The lesion is on the left lower leg. Cropped from a whole-body photographic skin survey; the tile spans about 15 mm. A male subject approximately 35 years of age:
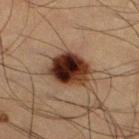Q: How was the tile lit?
A: cross-polarized illumination
Q: What did automated image analysis measure?
A: a lesion area of about 14 mm² and a shape eccentricity near 0.55; an average lesion color of about L≈28 a*≈19 b*≈24 (CIELAB), a lesion–skin lightness drop of about 20, and a normalized border contrast of about 17.5; border irregularity of about 2 on a 0–10 scale, internal color variation of about 10 on a 0–10 scale, and a peripheral color-asymmetry measure near 3.5
Q: What is the histopathologic diagnosis?
A: a dysplastic (Clark) nevus